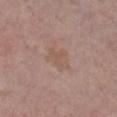Captured during whole-body skin photography for melanoma surveillance; the lesion was not biopsied. This is a white-light tile. On the leg. Measured at roughly 3.5 mm in maximum diameter. The patient is a female aged 63–67. A region of skin cropped from a whole-body photographic capture, roughly 15 mm wide.